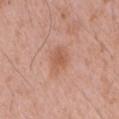{
  "biopsy_status": "not biopsied; imaged during a skin examination",
  "patient": {
    "sex": "male",
    "age_approx": 75
  },
  "image": {
    "source": "total-body photography crop",
    "field_of_view_mm": 15
  },
  "site": "mid back",
  "lighting": "white-light"
}